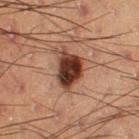The lesion was tiled from a total-body skin photograph and was not biopsied. On the right thigh. The total-body-photography lesion software estimated a mean CIELAB color near L≈30 a*≈21 b*≈24, about 19 CIELAB-L* units darker than the surrounding skin, and a normalized lesion–skin contrast near 16. And it measured border irregularity of about 2.5 on a 0–10 scale, a within-lesion color-variation index near 5.5/10, and a peripheral color-asymmetry measure near 1.5. The analysis additionally found a classifier nevus-likeness of about 100/100 and lesion-presence confidence of about 100/100. The tile uses cross-polarized illumination. A male patient aged 53 to 57. About 4.5 mm across. A 15 mm close-up tile from a total-body photography series done for melanoma screening.